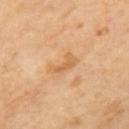notes: no biopsy performed (imaged during a skin exam)